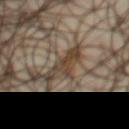workup = no biopsy performed (imaged during a skin exam)
patient = male, in their mid- to late 60s
illumination = cross-polarized
imaging modality = total-body-photography crop, ~15 mm field of view
lesion diameter = ≈4.5 mm
anatomic site = the chest